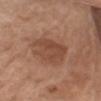Imaged during a routine full-body skin examination; the lesion was not biopsied and no histopathology is available.
An algorithmic analysis of the crop reported an eccentricity of roughly 0.7 and two-axis asymmetry of about 0.25. It also reported a mean CIELAB color near L≈47 a*≈22 b*≈30, a lesion–skin lightness drop of about 9, and a normalized border contrast of about 6.5. The software also gave a border-irregularity rating of about 3.5/10 and internal color variation of about 3 on a 0–10 scale. The software also gave lesion-presence confidence of about 100/100.
On the left upper arm.
Approximately 5.5 mm at its widest.
A region of skin cropped from a whole-body photographic capture, roughly 15 mm wide.
The patient is a female aged 73 to 77.